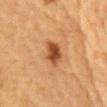Q: Is there a histopathology result?
A: catalogued during a skin exam; not biopsied
Q: Who is the patient?
A: male, in their mid- to late 80s or older
Q: Where on the body is the lesion?
A: the chest
Q: How was this image acquired?
A: ~15 mm tile from a whole-body skin photo
Q: What did automated image analysis measure?
A: an eccentricity of roughly 0.8 and two-axis asymmetry of about 0.2; a peripheral color-asymmetry measure near 1.5
Q: How large is the lesion?
A: about 3.5 mm